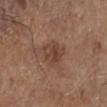  biopsy_status: not biopsied; imaged during a skin examination
  lesion_size:
    long_diameter_mm_approx: 3.5
  site: left lower leg
  patient:
    sex: male
    age_approx: 75
  image:
    source: total-body photography crop
    field_of_view_mm: 15